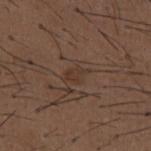image = ~15 mm crop, total-body skin-cancer survey
TBP lesion metrics = a footprint of about 3.5 mm², an eccentricity of roughly 0.75, and two-axis asymmetry of about 0.25; a classifier nevus-likeness of about 0/100 and lesion-presence confidence of about 95/100
diameter = about 3 mm
location = the chest
patient = male, in their 50s
lighting = white-light illumination